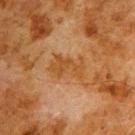Imaged during a routine full-body skin examination; the lesion was not biopsied and no histopathology is available. On the upper back. A male patient about 80 years old. The recorded lesion diameter is about 4.5 mm. The tile uses cross-polarized illumination. This image is a 15 mm lesion crop taken from a total-body photograph.